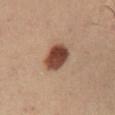Clinical impression: The lesion was photographed on a routine skin check and not biopsied; there is no pathology result. Clinical summary: The lesion is on the left lower leg. A male patient, aged around 55. The total-body-photography lesion software estimated a lesion area of about 9.5 mm², an eccentricity of roughly 0.55, and two-axis asymmetry of about 0.15. The software also gave a lesion color around L≈35 a*≈18 b*≈23 in CIELAB, about 15 CIELAB-L* units darker than the surrounding skin, and a normalized border contrast of about 12.5. The software also gave radial color variation of about 1.5. The analysis additionally found an automated nevus-likeness rating near 100 out of 100 and a detector confidence of about 100 out of 100 that the crop contains a lesion. A 15 mm close-up tile from a total-body photography series done for melanoma screening. This is a cross-polarized tile. The recorded lesion diameter is about 4 mm.